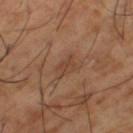Q: Is there a histopathology result?
A: catalogued during a skin exam; not biopsied
Q: How large is the lesion?
A: ~3 mm (longest diameter)
Q: Patient demographics?
A: male, about 65 years old
Q: What did automated image analysis measure?
A: a footprint of about 5 mm², an outline eccentricity of about 0.75 (0 = round, 1 = elongated), and two-axis asymmetry of about 0.4; a nevus-likeness score of about 0/100 and a lesion-detection confidence of about 100/100
Q: Lesion location?
A: the left thigh
Q: What is the imaging modality?
A: ~15 mm tile from a whole-body skin photo
Q: How was the tile lit?
A: cross-polarized illumination